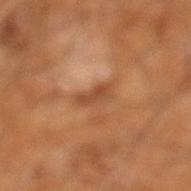This lesion was catalogued during total-body skin photography and was not selected for biopsy.
A male patient, in their 60s.
Cropped from a whole-body photographic skin survey; the tile spans about 15 mm.
From the right leg.
About 3 mm across.